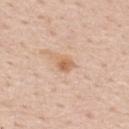workup: no biopsy performed (imaged during a skin exam)
acquisition: total-body-photography crop, ~15 mm field of view
body site: the back
patient: male, aged 58 to 62